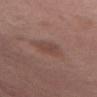The lesion was tiled from a total-body skin photograph and was not biopsied.
A 15 mm crop from a total-body photograph taken for skin-cancer surveillance.
Automated image analysis of the tile measured an average lesion color of about L≈45 a*≈20 b*≈24 (CIELAB) and about 7 CIELAB-L* units darker than the surrounding skin. The software also gave a border-irregularity rating of about 2/10 and radial color variation of about 1.
This is a white-light tile.
Longest diameter approximately 3 mm.
A female patient, roughly 40 years of age.
The lesion is located on the abdomen.